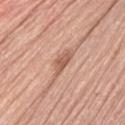Captured during whole-body skin photography for melanoma surveillance; the lesion was not biopsied.
The lesion is on the left thigh.
The lesion-visualizer software estimated an area of roughly 4 mm², an outline eccentricity of about 0.9 (0 = round, 1 = elongated), and a symmetry-axis asymmetry near 0.45. The analysis additionally found a mean CIELAB color near L≈58 a*≈23 b*≈30, a lesion–skin lightness drop of about 11, and a normalized lesion–skin contrast near 7.5. And it measured border irregularity of about 5 on a 0–10 scale and a peripheral color-asymmetry measure near 1.
The patient is a female aged approximately 60.
A region of skin cropped from a whole-body photographic capture, roughly 15 mm wide.
Longest diameter approximately 4 mm.